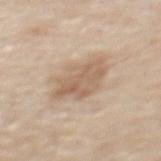Notes:
– workup — no biopsy performed (imaged during a skin exam)
– imaging modality — 15 mm crop, total-body photography
– location — the mid back
– subject — female, aged 63 to 67
– tile lighting — white-light